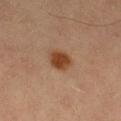{"automated_metrics": {"cielab_L": 35, "cielab_a": 19, "cielab_b": 28, "vs_skin_darker_L": 10.0, "border_irregularity_0_10": 2.0}, "patient": {"sex": "male", "age_approx": 70}, "image": {"source": "total-body photography crop", "field_of_view_mm": 15}, "site": "right thigh", "lighting": "cross-polarized"}